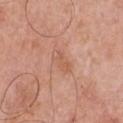The total-body-photography lesion software estimated a shape eccentricity near 0.9 and a symmetry-axis asymmetry near 0.3. The analysis additionally found a within-lesion color-variation index near 1/10. Located on the chest. A 15 mm close-up extracted from a 3D total-body photography capture. Imaged with white-light lighting. A male subject in their 70s. Approximately 3 mm at its widest.